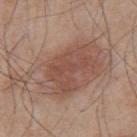Part of a total-body skin-imaging series; this lesion was reviewed on a skin check and was not flagged for biopsy. The lesion-visualizer software estimated a footprint of about 47 mm², an outline eccentricity of about 0.9 (0 = round, 1 = elongated), and two-axis asymmetry of about 0.35. The analysis additionally found an average lesion color of about L≈52 a*≈18 b*≈25 (CIELAB), roughly 9 lightness units darker than nearby skin, and a normalized lesion–skin contrast near 6.5. And it measured an automated nevus-likeness rating near 80 out of 100 and a lesion-detection confidence of about 100/100. Cropped from a whole-body photographic skin survey; the tile spans about 15 mm. Longest diameter approximately 12.5 mm. Imaged with white-light lighting. The lesion is located on the upper back. The subject is a male aged 53–57.